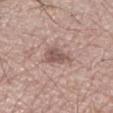Part of a total-body skin-imaging series; this lesion was reviewed on a skin check and was not flagged for biopsy.
A male subject about 65 years old.
The lesion is on the right upper arm.
A 15 mm close-up tile from a total-body photography series done for melanoma screening.